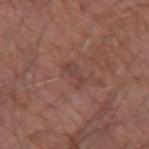A male subject, aged 48 to 52. The lesion is located on the left forearm. A 15 mm close-up extracted from a 3D total-body photography capture.